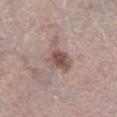Impression: Part of a total-body skin-imaging series; this lesion was reviewed on a skin check and was not flagged for biopsy.